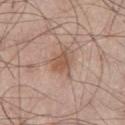Clinical impression:
The lesion was photographed on a routine skin check and not biopsied; there is no pathology result.
Acquisition and patient details:
On the left thigh. An algorithmic analysis of the crop reported a lesion area of about 7 mm², an outline eccentricity of about 0.75 (0 = round, 1 = elongated), and a shape-asymmetry score of about 0.4 (0 = symmetric). The software also gave border irregularity of about 4.5 on a 0–10 scale and a color-variation rating of about 2.5/10. The analysis additionally found a classifier nevus-likeness of about 50/100 and a lesion-detection confidence of about 100/100. Imaged with white-light lighting. Measured at roughly 4 mm in maximum diameter. A lesion tile, about 15 mm wide, cut from a 3D total-body photograph. A male patient, approximately 60 years of age.